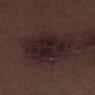Part of a total-body skin-imaging series; this lesion was reviewed on a skin check and was not flagged for biopsy. A male subject roughly 70 years of age. A 15 mm close-up extracted from a 3D total-body photography capture. The lesion is located on the leg. Captured under white-light illumination.